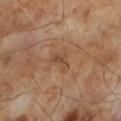<tbp_lesion>
<lighting>cross-polarized</lighting>
<automated_metrics>
  <area_mm2_approx>3.0</area_mm2_approx>
  <eccentricity>0.9</eccentricity>
  <vs_skin_darker_L>7.0</vs_skin_darker_L>
  <color_variation_0_10>0.5</color_variation_0_10>
</automated_metrics>
<patient>
  <sex>male</sex>
  <age_approx>65</age_approx>
</patient>
<lesion_size>
  <long_diameter_mm_approx>3.0</long_diameter_mm_approx>
</lesion_size>
<image>
  <source>total-body photography crop</source>
  <field_of_view_mm>15</field_of_view_mm>
</image>
</tbp_lesion>